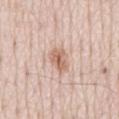Notes:
* lesion diameter: ~3 mm (longest diameter)
* image: 15 mm crop, total-body photography
* location: the back
* patient: male, approximately 80 years of age
* tile lighting: white-light